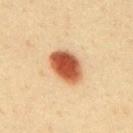Impression:
Recorded during total-body skin imaging; not selected for excision or biopsy.
Background:
Located on the upper back. A 15 mm close-up tile from a total-body photography series done for melanoma screening. Automated image analysis of the tile measured roughly 20 lightness units darker than nearby skin and a normalized lesion–skin contrast near 13. A male subject, aged 38–42. The lesion's longest dimension is about 4.5 mm.